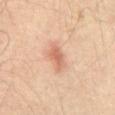Findings:
• workup · total-body-photography surveillance lesion; no biopsy
• subject · male, aged 53–57
• tile lighting · cross-polarized
• image · ~15 mm tile from a whole-body skin photo
• lesion size · ~3.5 mm (longest diameter)
• site · the abdomen